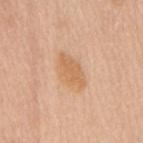A female patient, aged approximately 70. On the back. A roughly 15 mm field-of-view crop from a total-body skin photograph.